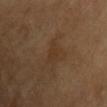Recorded during total-body skin imaging; not selected for excision or biopsy. Longest diameter approximately 2.5 mm. Captured under cross-polarized illumination. Located on the chest. The lesion-visualizer software estimated a mean CIELAB color near L≈32 a*≈15 b*≈27, roughly 4 lightness units darker than nearby skin, and a lesion-to-skin contrast of about 5 (normalized; higher = more distinct). The software also gave a classifier nevus-likeness of about 0/100. A lesion tile, about 15 mm wide, cut from a 3D total-body photograph. A male patient aged 58 to 62.